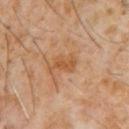Clinical impression: The lesion was photographed on a routine skin check and not biopsied; there is no pathology result. Image and clinical context: A 15 mm close-up extracted from a 3D total-body photography capture. Automated tile analysis of the lesion measured an average lesion color of about L≈49 a*≈21 b*≈36 (CIELAB) and a lesion–skin lightness drop of about 8. It also reported a border-irregularity index near 5.5/10, a color-variation rating of about 1.5/10, and radial color variation of about 0.5. It also reported a nevus-likeness score of about 0/100. A male subject, aged approximately 60. Located on the chest.